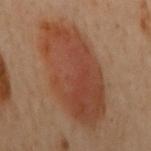Q: Is there a histopathology result?
A: total-body-photography surveillance lesion; no biopsy
Q: How large is the lesion?
A: ≈11.5 mm
Q: How was the tile lit?
A: cross-polarized illumination
Q: Patient demographics?
A: female, aged 58 to 62
Q: What did automated image analysis measure?
A: an automated nevus-likeness rating near 100 out of 100 and a detector confidence of about 100 out of 100 that the crop contains a lesion
Q: Where on the body is the lesion?
A: the back
Q: How was this image acquired?
A: 15 mm crop, total-body photography